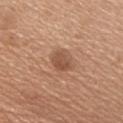workup: total-body-photography surveillance lesion; no biopsy
lesion diameter: about 3 mm
site: the chest
subject: male, in their 60s
imaging modality: total-body-photography crop, ~15 mm field of view